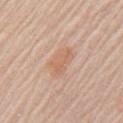Recorded during total-body skin imaging; not selected for excision or biopsy.
Cropped from a total-body skin-imaging series; the visible field is about 15 mm.
An algorithmic analysis of the crop reported an area of roughly 7.5 mm², an outline eccentricity of about 0.75 (0 = round, 1 = elongated), and two-axis asymmetry of about 0.3.
A female patient, about 65 years old.
The lesion's longest dimension is about 4 mm.
Captured under white-light illumination.
Located on the left upper arm.